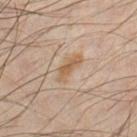notes — no biopsy performed (imaged during a skin exam)
image source — 15 mm crop, total-body photography
subject — male, about 35 years old
automated metrics — an area of roughly 5 mm² and two-axis asymmetry of about 0.35; a lesion–skin lightness drop of about 8 and a normalized border contrast of about 7; internal color variation of about 1.5 on a 0–10 scale and radial color variation of about 0.5; lesion-presence confidence of about 100/100
location — the chest
tile lighting — cross-polarized
lesion diameter — ≈4 mm